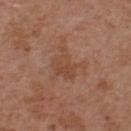Recorded during total-body skin imaging; not selected for excision or biopsy.
Located on the front of the torso.
The total-body-photography lesion software estimated an eccentricity of roughly 0.55 and a shape-asymmetry score of about 0.55 (0 = symmetric). The analysis additionally found a lesion color around L≈47 a*≈20 b*≈31 in CIELAB and a normalized lesion–skin contrast near 5.5. The analysis additionally found a detector confidence of about 100 out of 100 that the crop contains a lesion.
A close-up tile cropped from a whole-body skin photograph, about 15 mm across.
Captured under white-light illumination.
Approximately 5 mm at its widest.
The subject is a male aged 53–57.